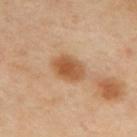Clinical impression:
The lesion was tiled from a total-body skin photograph and was not biopsied.
Context:
On the upper back. A 15 mm close-up tile from a total-body photography series done for melanoma screening. A female subject approximately 40 years of age.